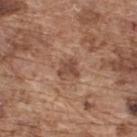Assessment: Imaged during a routine full-body skin examination; the lesion was not biopsied and no histopathology is available. Image and clinical context: Cropped from a total-body skin-imaging series; the visible field is about 15 mm. Imaged with white-light lighting. Located on the upper back. The subject is a male aged approximately 75. Measured at roughly 2.5 mm in maximum diameter.